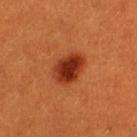workup=no biopsy performed (imaged during a skin exam); location=the left thigh; lighting=cross-polarized illumination; patient=female, aged approximately 30; image source=total-body-photography crop, ~15 mm field of view.